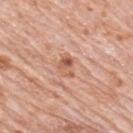biopsy status: no biopsy performed (imaged during a skin exam).